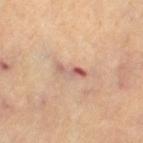Findings:
* acquisition — 15 mm crop, total-body photography
* lesion diameter — ≈3.5 mm
* patient — female, aged 63–67
* automated metrics — a footprint of about 3.5 mm², a shape eccentricity near 0.95, and a symmetry-axis asymmetry near 0.35; a lesion color around L≈57 a*≈21 b*≈26 in CIELAB, about 10 CIELAB-L* units darker than the surrounding skin, and a normalized lesion–skin contrast near 7.5; border irregularity of about 4.5 on a 0–10 scale and internal color variation of about 2 on a 0–10 scale
* lighting — cross-polarized
* body site — the left thigh